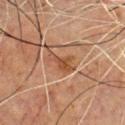Q: Was a biopsy performed?
A: catalogued during a skin exam; not biopsied
Q: What is the imaging modality?
A: ~15 mm crop, total-body skin-cancer survey
Q: Who is the patient?
A: male, approximately 50 years of age
Q: Where on the body is the lesion?
A: the chest